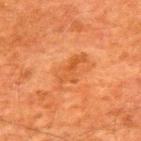Findings:
• notes: imaged on a skin check; not biopsied
• location: the upper back
• image source: 15 mm crop, total-body photography
• automated metrics: a lesion color around L≈44 a*≈26 b*≈38 in CIELAB, a lesion–skin lightness drop of about 6, and a lesion-to-skin contrast of about 5.5 (normalized; higher = more distinct); a classifier nevus-likeness of about 0/100 and a detector confidence of about 100 out of 100 that the crop contains a lesion
• lesion size: ≈4.5 mm
• subject: male, aged 58 to 62